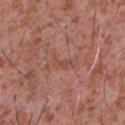notes=total-body-photography surveillance lesion; no biopsy
anatomic site=the chest
lesion diameter=~2.5 mm (longest diameter)
image=~15 mm tile from a whole-body skin photo
subject=male, aged 43 to 47